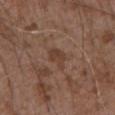Case summary:
* notes: catalogued during a skin exam; not biopsied
* subject: male, aged approximately 75
* body site: the abdomen
* acquisition: total-body-photography crop, ~15 mm field of view
* automated metrics: two-axis asymmetry of about 0.3; an average lesion color of about L≈39 a*≈18 b*≈26 (CIELAB), roughly 7 lightness units darker than nearby skin, and a normalized lesion–skin contrast near 6.5; a border-irregularity rating of about 3/10 and a within-lesion color-variation index near 2/10; a classifier nevus-likeness of about 0/100 and a detector confidence of about 100 out of 100 that the crop contains a lesion
* size: ~2.5 mm (longest diameter)